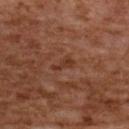| field | value |
|---|---|
| workup | catalogued during a skin exam; not biopsied |
| patient | female, in their 60s |
| image source | ~15 mm tile from a whole-body skin photo |
| size | ≈3 mm |
| site | the back |
| lighting | cross-polarized illumination |